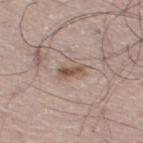Clinical impression:
Recorded during total-body skin imaging; not selected for excision or biopsy.
Acquisition and patient details:
About 3 mm across. The lesion is on the left leg. A male subject about 70 years old. Captured under white-light illumination. Cropped from a total-body skin-imaging series; the visible field is about 15 mm. The total-body-photography lesion software estimated a lesion area of about 4 mm² and a shape-asymmetry score of about 0.25 (0 = symmetric). And it measured an average lesion color of about L≈53 a*≈17 b*≈27 (CIELAB), about 11 CIELAB-L* units darker than the surrounding skin, and a normalized border contrast of about 8.5. It also reported a border-irregularity rating of about 3/10 and radial color variation of about 1. The analysis additionally found an automated nevus-likeness rating near 85 out of 100.